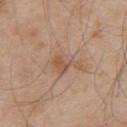biopsy status: total-body-photography surveillance lesion; no biopsy | lighting: white-light illumination | subject: male, aged approximately 55 | image: 15 mm crop, total-body photography | TBP lesion metrics: an automated nevus-likeness rating near 0 out of 100 | lesion diameter: ≈5.5 mm | location: the upper back.